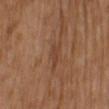{"biopsy_status": "not biopsied; imaged during a skin examination"}